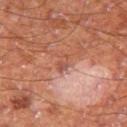The lesion was tiled from a total-body skin photograph and was not biopsied. A 15 mm close-up tile from a total-body photography series done for melanoma screening. The total-body-photography lesion software estimated a lesion area of about 2.5 mm², an eccentricity of roughly 0.85, and a shape-asymmetry score of about 0.3 (0 = symmetric). The software also gave a border-irregularity rating of about 3/10, a within-lesion color-variation index near 1/10, and a peripheral color-asymmetry measure near 0. About 2.5 mm across. A male patient, approximately 60 years of age. The lesion is located on the right thigh.